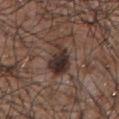Q: Was this lesion biopsied?
A: no biopsy performed (imaged during a skin exam)
Q: What lighting was used for the tile?
A: white-light
Q: What kind of image is this?
A: ~15 mm tile from a whole-body skin photo
Q: Patient demographics?
A: male, about 55 years old
Q: Automated lesion metrics?
A: a normalized lesion–skin contrast near 12; an automated nevus-likeness rating near 45 out of 100
Q: Where on the body is the lesion?
A: the chest